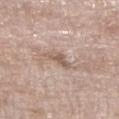notes=no biopsy performed (imaged during a skin exam)